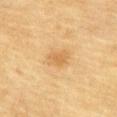Clinical impression:
No biopsy was performed on this lesion — it was imaged during a full skin examination and was not determined to be concerning.
Image and clinical context:
The tile uses cross-polarized illumination. Cropped from a whole-body photographic skin survey; the tile spans about 15 mm. A male subject aged 83 or over. Measured at roughly 2.5 mm in maximum diameter. The lesion is located on the front of the torso.